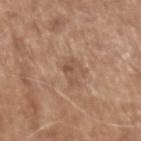Background:
The tile uses white-light illumination. The lesion's longest dimension is about 3 mm. From the left upper arm. The total-body-photography lesion software estimated a footprint of about 3 mm², an eccentricity of roughly 0.9, and two-axis asymmetry of about 0.55. It also reported border irregularity of about 6 on a 0–10 scale, a within-lesion color-variation index near 1/10, and a peripheral color-asymmetry measure near 0.5. The analysis additionally found an automated nevus-likeness rating near 0 out of 100 and a lesion-detection confidence of about 100/100. Cropped from a whole-body photographic skin survey; the tile spans about 15 mm. A male subject aged 73 to 77.